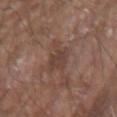Captured during whole-body skin photography for melanoma surveillance; the lesion was not biopsied. The lesion is located on the right upper arm. Cropped from a total-body skin-imaging series; the visible field is about 15 mm. About 4 mm across. The patient is a male aged around 80. This is a white-light tile. The total-body-photography lesion software estimated an area of roughly 7 mm² and a shape-asymmetry score of about 0.4 (0 = symmetric). And it measured an average lesion color of about L≈41 a*≈18 b*≈23 (CIELAB), roughly 7 lightness units darker than nearby skin, and a normalized lesion–skin contrast near 6. It also reported a classifier nevus-likeness of about 0/100.